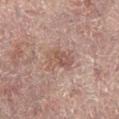Q: Is there a histopathology result?
A: no biopsy performed (imaged during a skin exam)
Q: Who is the patient?
A: female, aged 78 to 82
Q: How large is the lesion?
A: ≈4 mm
Q: Automated lesion metrics?
A: an eccentricity of roughly 0.75 and two-axis asymmetry of about 0.25
Q: Illumination type?
A: cross-polarized
Q: What kind of image is this?
A: 15 mm crop, total-body photography
Q: Lesion location?
A: the left leg